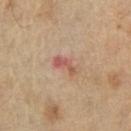The lesion was tiled from a total-body skin photograph and was not biopsied. The lesion-visualizer software estimated an area of roughly 4 mm², a shape eccentricity near 0.9, and a symmetry-axis asymmetry near 0.35. The analysis additionally found border irregularity of about 4 on a 0–10 scale, internal color variation of about 1.5 on a 0–10 scale, and radial color variation of about 0. The analysis additionally found a classifier nevus-likeness of about 0/100 and a lesion-detection confidence of about 100/100. A region of skin cropped from a whole-body photographic capture, roughly 15 mm wide. A male subject, aged 68–72. About 3.5 mm across. The lesion is located on the front of the torso.